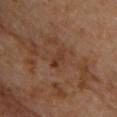notes: imaged on a skin check; not biopsied | image: ~15 mm tile from a whole-body skin photo | patient: male, approximately 60 years of age | anatomic site: the front of the torso | illumination: cross-polarized | size: ≈2.5 mm | automated lesion analysis: an area of roughly 3 mm², an eccentricity of roughly 0.85, and a symmetry-axis asymmetry near 0.3; an average lesion color of about L≈34 a*≈20 b*≈29 (CIELAB), a lesion–skin lightness drop of about 6, and a normalized border contrast of about 6.5; border irregularity of about 3 on a 0–10 scale, a within-lesion color-variation index near 1/10, and peripheral color asymmetry of about 0.5.